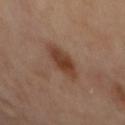No biopsy was performed on this lesion — it was imaged during a full skin examination and was not determined to be concerning. The lesion is located on the mid back. This image is a 15 mm lesion crop taken from a total-body photograph.